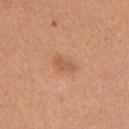workup: no biopsy performed (imaged during a skin exam); acquisition: 15 mm crop, total-body photography; tile lighting: white-light; subject: female, about 40 years old; anatomic site: the left upper arm.